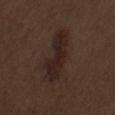This lesion was catalogued during total-body skin photography and was not selected for biopsy.
A male subject, aged 68–72.
The lesion-visualizer software estimated a lesion area of about 13 mm² and two-axis asymmetry of about 0.3. It also reported an average lesion color of about L≈21 a*≈15 b*≈18 (CIELAB), roughly 7 lightness units darker than nearby skin, and a lesion-to-skin contrast of about 9 (normalized; higher = more distinct). It also reported a nevus-likeness score of about 80/100 and lesion-presence confidence of about 100/100.
Longest diameter approximately 7.5 mm.
Located on the left upper arm.
A region of skin cropped from a whole-body photographic capture, roughly 15 mm wide.